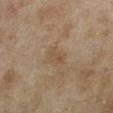Captured during whole-body skin photography for melanoma surveillance; the lesion was not biopsied. Longest diameter approximately 2.5 mm. Cropped from a whole-body photographic skin survey; the tile spans about 15 mm. Located on the left lower leg. A female patient about 60 years old. Automated image analysis of the tile measured a classifier nevus-likeness of about 0/100 and a detector confidence of about 100 out of 100 that the crop contains a lesion. The tile uses cross-polarized illumination.